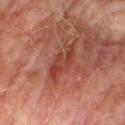| key | value |
|---|---|
| biopsy status | total-body-photography surveillance lesion; no biopsy |
| illumination | cross-polarized |
| TBP lesion metrics | an average lesion color of about L≈34 a*≈24 b*≈25 (CIELAB), roughly 7 lightness units darker than nearby skin, and a normalized border contrast of about 7 |
| subject | male, in their mid-70s |
| lesion size | ≈5.5 mm |
| body site | the right thigh |
| image source | total-body-photography crop, ~15 mm field of view |